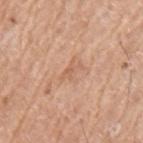<lesion>
<biopsy_status>not biopsied; imaged during a skin examination</biopsy_status>
</lesion>